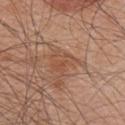Imaged during a routine full-body skin examination; the lesion was not biopsied and no histopathology is available. From the upper back. Captured under white-light illumination. A male subject approximately 45 years of age. Cropped from a total-body skin-imaging series; the visible field is about 15 mm. The recorded lesion diameter is about 3.5 mm.